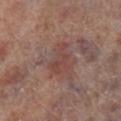workup = imaged on a skin check; not biopsied | location = the right lower leg | subject = male, aged around 70 | lighting = white-light illumination | acquisition = ~15 mm tile from a whole-body skin photo | automated lesion analysis = a lesion color around L≈44 a*≈21 b*≈22 in CIELAB, a lesion–skin lightness drop of about 7, and a normalized lesion–skin contrast near 5.5.